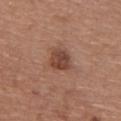Assessment: Captured during whole-body skin photography for melanoma surveillance; the lesion was not biopsied. Image and clinical context: The lesion is located on the chest. The patient is a male aged around 55. A close-up tile cropped from a whole-body skin photograph, about 15 mm across. Imaged with white-light lighting. The lesion's longest dimension is about 3 mm. The total-body-photography lesion software estimated a footprint of about 6.5 mm², an eccentricity of roughly 0.35, and two-axis asymmetry of about 0.25. The analysis additionally found a lesion color around L≈44 a*≈22 b*≈27 in CIELAB and about 11 CIELAB-L* units darker than the surrounding skin. And it measured an automated nevus-likeness rating near 55 out of 100 and a lesion-detection confidence of about 100/100.